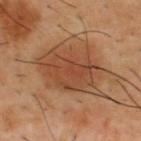patient = male, aged around 40
TBP lesion metrics = a lesion area of about 27 mm², a shape eccentricity near 0.75, and a symmetry-axis asymmetry near 0.25; an average lesion color of about L≈45 a*≈23 b*≈33 (CIELAB) and a normalized border contrast of about 7.5
tile lighting = cross-polarized
diameter = ~7.5 mm (longest diameter)
body site = the upper back
image = total-body-photography crop, ~15 mm field of view